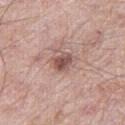Imaged during a routine full-body skin examination; the lesion was not biopsied and no histopathology is available. A male subject, in their mid-60s. A 15 mm crop from a total-body photograph taken for skin-cancer surveillance. From the right thigh.